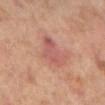follow-up = no biopsy performed (imaged during a skin exam)
image = 15 mm crop, total-body photography
subject = male, roughly 70 years of age
anatomic site = the left lower leg
diameter = ~4 mm (longest diameter)
automated metrics = an eccentricity of roughly 0.75 and a symmetry-axis asymmetry near 0.4